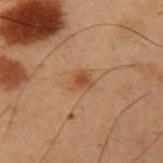The lesion was photographed on a routine skin check and not biopsied; there is no pathology result.
Imaged with cross-polarized lighting.
A male patient, approximately 55 years of age.
The lesion is located on the left upper arm.
The total-body-photography lesion software estimated a lesion area of about 4 mm² and an outline eccentricity of about 0.6 (0 = round, 1 = elongated).
A 15 mm crop from a total-body photograph taken for skin-cancer surveillance.